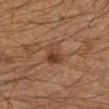{
  "biopsy_status": "not biopsied; imaged during a skin examination",
  "patient": {
    "sex": "male",
    "age_approx": 50
  },
  "image": {
    "source": "total-body photography crop",
    "field_of_view_mm": 15
  },
  "lighting": "cross-polarized",
  "lesion_size": {
    "long_diameter_mm_approx": 3.5
  },
  "automated_metrics": {
    "area_mm2_approx": 6.5,
    "shape_asymmetry": 0.35,
    "cielab_L": 32,
    "cielab_a": 17,
    "cielab_b": 25,
    "vs_skin_darker_L": 7.0
  },
  "site": "left forearm"
}